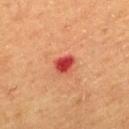workup = catalogued during a skin exam; not biopsied | size = about 2.5 mm | subject = male, aged 58 to 62 | site = the mid back | image source = ~15 mm tile from a whole-body skin photo | automated metrics = an area of roughly 4.5 mm², a shape eccentricity near 0.65, and two-axis asymmetry of about 0.25; border irregularity of about 2 on a 0–10 scale, a color-variation rating of about 4/10, and radial color variation of about 1; a classifier nevus-likeness of about 0/100 and a lesion-detection confidence of about 100/100 | lighting = cross-polarized.